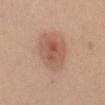follow-up = no biopsy performed (imaged during a skin exam)
image source = ~15 mm crop, total-body skin-cancer survey
illumination = white-light
subject = female, aged around 35
body site = the back